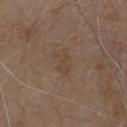This lesion was catalogued during total-body skin photography and was not selected for biopsy. A close-up tile cropped from a whole-body skin photograph, about 15 mm across. The recorded lesion diameter is about 3 mm. The lesion-visualizer software estimated an area of roughly 4 mm², a shape eccentricity near 0.85, and a symmetry-axis asymmetry near 0.3. It also reported a within-lesion color-variation index near 1/10 and peripheral color asymmetry of about 0.5. It also reported a lesion-detection confidence of about 100/100. A male subject, aged around 80. From the front of the torso.